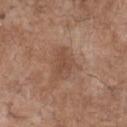Recorded during total-body skin imaging; not selected for excision or biopsy. The subject is a male approximately 70 years of age. From the chest. Cropped from a whole-body photographic skin survey; the tile spans about 15 mm.